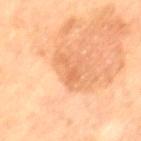Assessment: Part of a total-body skin-imaging series; this lesion was reviewed on a skin check and was not flagged for biopsy. Clinical summary: The lesion-visualizer software estimated a footprint of about 4.5 mm² and a shape-asymmetry score of about 0.65 (0 = symmetric). The analysis additionally found border irregularity of about 7.5 on a 0–10 scale and a peripheral color-asymmetry measure near 0.5. The recorded lesion diameter is about 4 mm. This image is a 15 mm lesion crop taken from a total-body photograph. From the left thigh. A female subject, aged 68 to 72. This is a cross-polarized tile.